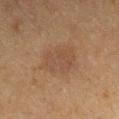Cropped from a whole-body photographic skin survey; the tile spans about 15 mm. The tile uses cross-polarized illumination. The lesion is located on the left upper arm. Automated image analysis of the tile measured a symmetry-axis asymmetry near 0.2. It also reported internal color variation of about 2.5 on a 0–10 scale and a peripheral color-asymmetry measure near 1. The software also gave a classifier nevus-likeness of about 0/100 and lesion-presence confidence of about 100/100. About 4 mm across. The patient is a male aged around 65.